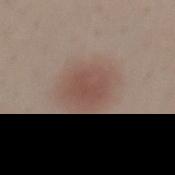* biopsy status — catalogued during a skin exam; not biopsied
* image source — 15 mm crop, total-body photography
* subject — male, about 30 years old
* location — the mid back